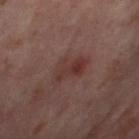Impression:
This lesion was catalogued during total-body skin photography and was not selected for biopsy.
Image and clinical context:
A lesion tile, about 15 mm wide, cut from a 3D total-body photograph. The subject is a female approximately 55 years of age. Located on the right thigh. Imaged with cross-polarized lighting.